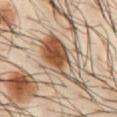Acquisition and patient details: A male patient, in their 40s. The tile uses cross-polarized illumination. The lesion is located on the abdomen. An algorithmic analysis of the crop reported a footprint of about 17 mm², an outline eccentricity of about 0.9 (0 = round, 1 = elongated), and a symmetry-axis asymmetry near 0.5. The software also gave a lesion–skin lightness drop of about 15 and a lesion-to-skin contrast of about 11 (normalized; higher = more distinct). The analysis additionally found a nevus-likeness score of about 100/100. Measured at roughly 8.5 mm in maximum diameter. A 15 mm close-up extracted from a 3D total-body photography capture.